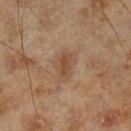{
  "biopsy_status": "not biopsied; imaged during a skin examination",
  "site": "left lower leg",
  "image": {
    "source": "total-body photography crop",
    "field_of_view_mm": 15
  },
  "automated_metrics": {
    "area_mm2_approx": 4.0,
    "eccentricity": 0.85,
    "shape_asymmetry": 0.3,
    "cielab_L": 45,
    "cielab_a": 19,
    "cielab_b": 31,
    "vs_skin_darker_L": 8.0,
    "vs_skin_contrast_norm": 6.5
  },
  "lesion_size": {
    "long_diameter_mm_approx": 3.0
  },
  "patient": {
    "sex": "male",
    "age_approx": 70
  },
  "lighting": "cross-polarized"
}